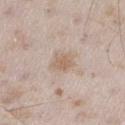From the right thigh. A male patient, about 45 years old. Captured under white-light illumination. Longest diameter approximately 2.5 mm. A roughly 15 mm field-of-view crop from a total-body skin photograph.